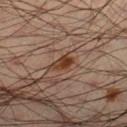Clinical impression:
Part of a total-body skin-imaging series; this lesion was reviewed on a skin check and was not flagged for biopsy.
Acquisition and patient details:
Measured at roughly 2.5 mm in maximum diameter. A 15 mm crop from a total-body photograph taken for skin-cancer surveillance. A male patient, in their mid- to late 30s. Automated tile analysis of the lesion measured a lesion color around L≈34 a*≈20 b*≈28 in CIELAB and about 10 CIELAB-L* units darker than the surrounding skin. And it measured border irregularity of about 2.5 on a 0–10 scale, a color-variation rating of about 2.5/10, and a peripheral color-asymmetry measure near 1. The software also gave an automated nevus-likeness rating near 85 out of 100. The lesion is located on the right lower leg. This is a cross-polarized tile.